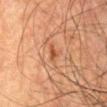Q: Is there a histopathology result?
A: imaged on a skin check; not biopsied
Q: Who is the patient?
A: male, approximately 80 years of age
Q: What is the anatomic site?
A: the abdomen
Q: What kind of image is this?
A: ~15 mm tile from a whole-body skin photo
Q: Lesion size?
A: ~2.5 mm (longest diameter)